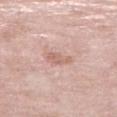Part of a total-body skin-imaging series; this lesion was reviewed on a skin check and was not flagged for biopsy. Located on the right lower leg. The lesion's longest dimension is about 3 mm. The tile uses white-light illumination. A lesion tile, about 15 mm wide, cut from a 3D total-body photograph. A female patient, about 70 years old.